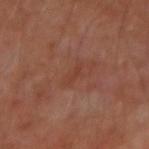Captured during whole-body skin photography for melanoma surveillance; the lesion was not biopsied.
The patient is a male roughly 50 years of age.
This is a cross-polarized tile.
Cropped from a total-body skin-imaging series; the visible field is about 15 mm.
The lesion is on the left arm.